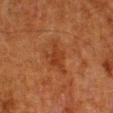Recorded during total-body skin imaging; not selected for excision or biopsy. Imaged with cross-polarized lighting. A lesion tile, about 15 mm wide, cut from a 3D total-body photograph. The lesion's longest dimension is about 3 mm. Located on the leg. A male patient about 80 years old. The lesion-visualizer software estimated a lesion color around L≈30 a*≈23 b*≈31 in CIELAB and a lesion-to-skin contrast of about 6 (normalized; higher = more distinct). The analysis additionally found internal color variation of about 1.5 on a 0–10 scale and a peripheral color-asymmetry measure near 0.5. The analysis additionally found a classifier nevus-likeness of about 0/100 and a lesion-detection confidence of about 100/100.